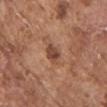Clinical impression: Part of a total-body skin-imaging series; this lesion was reviewed on a skin check and was not flagged for biopsy. Background: Located on the chest. A male subject, aged 73–77. A close-up tile cropped from a whole-body skin photograph, about 15 mm across.